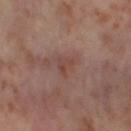follow-up: catalogued during a skin exam; not biopsied
tile lighting: cross-polarized illumination
body site: the left thigh
lesion size: ~2.5 mm (longest diameter)
imaging modality: ~15 mm tile from a whole-body skin photo
automated lesion analysis: a lesion color around L≈44 a*≈20 b*≈24 in CIELAB, roughly 6 lightness units darker than nearby skin, and a normalized border contrast of about 5.5; border irregularity of about 4.5 on a 0–10 scale
patient: female, in their mid- to late 50s